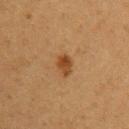Part of a total-body skin-imaging series; this lesion was reviewed on a skin check and was not flagged for biopsy.
Located on the left upper arm.
Automated tile analysis of the lesion measured a footprint of about 4 mm², an eccentricity of roughly 0.65, and a shape-asymmetry score of about 0.2 (0 = symmetric). The software also gave a within-lesion color-variation index near 3.5/10 and a peripheral color-asymmetry measure near 1. And it measured a classifier nevus-likeness of about 95/100 and a lesion-detection confidence of about 100/100.
Measured at roughly 2.5 mm in maximum diameter.
A roughly 15 mm field-of-view crop from a total-body skin photograph.
A female subject in their 40s.